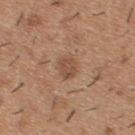workup = catalogued during a skin exam; not biopsied | lighting = white-light illumination | image = 15 mm crop, total-body photography | subject = male, roughly 40 years of age | anatomic site = the back | lesion diameter = about 2.5 mm.